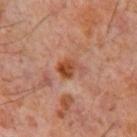Q: Was a biopsy performed?
A: no biopsy performed (imaged during a skin exam)
Q: How was this image acquired?
A: ~15 mm crop, total-body skin-cancer survey
Q: Lesion location?
A: the chest
Q: Automated lesion metrics?
A: a lesion color around L≈44 a*≈25 b*≈33 in CIELAB, roughly 11 lightness units darker than nearby skin, and a lesion-to-skin contrast of about 10 (normalized; higher = more distinct); border irregularity of about 3 on a 0–10 scale, a color-variation rating of about 4/10, and peripheral color asymmetry of about 1.5
Q: What lighting was used for the tile?
A: cross-polarized illumination
Q: What are the patient's age and sex?
A: male, aged 58–62
Q: What is the lesion's diameter?
A: ~3 mm (longest diameter)